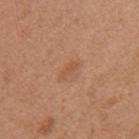Imaged during a routine full-body skin examination; the lesion was not biopsied and no histopathology is available. A close-up tile cropped from a whole-body skin photograph, about 15 mm across. Longest diameter approximately 3 mm. The tile uses white-light illumination. The subject is a female aged around 40. From the mid back.